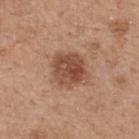Image and clinical context:
A male subject about 65 years old. The recorded lesion diameter is about 4 mm. Located on the back. A close-up tile cropped from a whole-body skin photograph, about 15 mm across. Automated tile analysis of the lesion measured a color-variation rating of about 5/10. Imaged with white-light lighting.